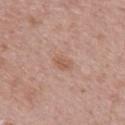{
  "biopsy_status": "not biopsied; imaged during a skin examination",
  "lighting": "white-light",
  "automated_metrics": {
    "cielab_L": 57,
    "cielab_a": 21,
    "cielab_b": 29,
    "vs_skin_darker_L": 7.0,
    "vs_skin_contrast_norm": 5.5
  },
  "patient": {
    "sex": "male",
    "age_approx": 65
  },
  "image": {
    "source": "total-body photography crop",
    "field_of_view_mm": 15
  },
  "site": "back",
  "lesion_size": {
    "long_diameter_mm_approx": 2.5
  }
}